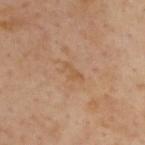Assessment: Part of a total-body skin-imaging series; this lesion was reviewed on a skin check and was not flagged for biopsy. Background: The subject is a male approximately 55 years of age. An algorithmic analysis of the crop reported a footprint of about 2 mm² and a shape eccentricity near 0.9. The analysis additionally found an average lesion color of about L≈54 a*≈20 b*≈35 (CIELAB), a lesion–skin lightness drop of about 6, and a normalized border contrast of about 5.5. The analysis additionally found a border-irregularity index near 6.5/10, a color-variation rating of about 0/10, and peripheral color asymmetry of about 0. And it measured a lesion-detection confidence of about 100/100. Imaged with cross-polarized lighting. On the upper back. The recorded lesion diameter is about 2.5 mm. This image is a 15 mm lesion crop taken from a total-body photograph.